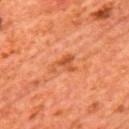follow-up: imaged on a skin check; not biopsied.